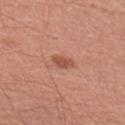biopsy status: total-body-photography surveillance lesion; no biopsy
subject: male, approximately 60 years of age
location: the right upper arm
automated metrics: an average lesion color of about L≈53 a*≈26 b*≈31 (CIELAB), about 10 CIELAB-L* units darker than the surrounding skin, and a lesion-to-skin contrast of about 7 (normalized; higher = more distinct); a border-irregularity rating of about 2.5/10, a within-lesion color-variation index near 2/10, and peripheral color asymmetry of about 0.5; a lesion-detection confidence of about 100/100
imaging modality: ~15 mm crop, total-body skin-cancer survey
tile lighting: white-light